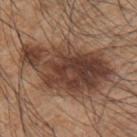This lesion was catalogued during total-body skin photography and was not selected for biopsy. The lesion's longest dimension is about 11 mm. A lesion tile, about 15 mm wide, cut from a 3D total-body photograph. The tile uses white-light illumination. From the arm. A male subject, roughly 45 years of age.